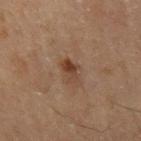Clinical impression:
This lesion was catalogued during total-body skin photography and was not selected for biopsy.
Background:
An algorithmic analysis of the crop reported border irregularity of about 2.5 on a 0–10 scale, internal color variation of about 5.5 on a 0–10 scale, and radial color variation of about 2. The analysis additionally found a classifier nevus-likeness of about 65/100 and a detector confidence of about 100 out of 100 that the crop contains a lesion. A close-up tile cropped from a whole-body skin photograph, about 15 mm across. A male patient about 70 years old. The lesion is on the right thigh. The lesion's longest dimension is about 3 mm. This is a cross-polarized tile.